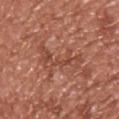{
  "biopsy_status": "not biopsied; imaged during a skin examination",
  "image": {
    "source": "total-body photography crop",
    "field_of_view_mm": 15
  },
  "patient": {
    "sex": "male",
    "age_approx": 55
  },
  "lesion_size": {
    "long_diameter_mm_approx": 6.0
  },
  "automated_metrics": {
    "area_mm2_approx": 9.0,
    "eccentricity": 0.95,
    "shape_asymmetry": 0.55,
    "border_irregularity_0_10": 9.0,
    "color_variation_0_10": 3.5
  },
  "lighting": "white-light",
  "site": "upper back"
}